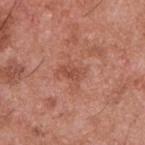Findings:
– follow-up · total-body-photography surveillance lesion; no biopsy
– patient · male, about 55 years old
– anatomic site · the upper back
– size · about 3 mm
– image · 15 mm crop, total-body photography